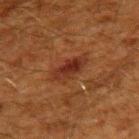Notes:
• workup — no biopsy performed (imaged during a skin exam)
• patient — male, roughly 60 years of age
• imaging modality — ~15 mm tile from a whole-body skin photo
• tile lighting — cross-polarized illumination
• TBP lesion metrics — a lesion color around L≈25 a*≈21 b*≈25 in CIELAB and about 7 CIELAB-L* units darker than the surrounding skin; an automated nevus-likeness rating near 45 out of 100 and a lesion-detection confidence of about 100/100
• body site — the upper back